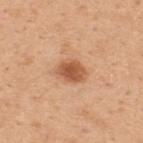Context: A male subject, aged around 50. On the upper back. This image is a 15 mm lesion crop taken from a total-body photograph. The lesion-visualizer software estimated an area of roughly 6.5 mm² and a shape eccentricity near 0.65. And it measured a classifier nevus-likeness of about 95/100 and a lesion-detection confidence of about 100/100. Measured at roughly 3.5 mm in maximum diameter.